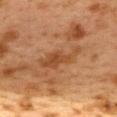notes: imaged on a skin check; not biopsied | anatomic site: the upper back | lesion diameter: about 5.5 mm | subject: female, roughly 40 years of age | tile lighting: cross-polarized | automated metrics: border irregularity of about 6 on a 0–10 scale, internal color variation of about 2.5 on a 0–10 scale, and a peripheral color-asymmetry measure near 1; a classifier nevus-likeness of about 0/100 and a lesion-detection confidence of about 100/100 | acquisition: 15 mm crop, total-body photography.